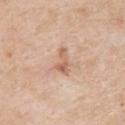Case summary:
- follow-up · catalogued during a skin exam; not biopsied
- subject · male, roughly 85 years of age or older
- TBP lesion metrics · a shape eccentricity near 0.85 and two-axis asymmetry of about 0.55
- tile lighting · white-light
- image source · 15 mm crop, total-body photography
- location · the upper back